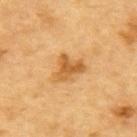follow-up=total-body-photography surveillance lesion; no biopsy | tile lighting=cross-polarized | lesion size=≈4 mm | location=the upper back | patient=male, roughly 85 years of age or older | imaging modality=~15 mm tile from a whole-body skin photo.